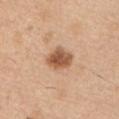biopsy status = no biopsy performed (imaged during a skin exam) | site = the left upper arm | subject = male, aged 38–42 | acquisition = ~15 mm tile from a whole-body skin photo | lesion diameter = ~3.5 mm (longest diameter) | image-analysis metrics = a lesion color around L≈55 a*≈22 b*≈34 in CIELAB and a lesion–skin lightness drop of about 15; a border-irregularity index near 1.5/10, internal color variation of about 4.5 on a 0–10 scale, and radial color variation of about 1.5.